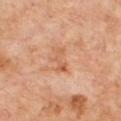workup: catalogued during a skin exam; not biopsied
TBP lesion metrics: a within-lesion color-variation index near 0/10 and radial color variation of about 0
imaging modality: ~15 mm crop, total-body skin-cancer survey
site: the chest
lighting: cross-polarized
lesion diameter: ≈3.5 mm
patient: male, in their 70s